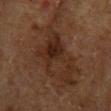<record>
  <biopsy_status>not biopsied; imaged during a skin examination</biopsy_status>
  <lighting>cross-polarized</lighting>
  <lesion_size>
    <long_diameter_mm_approx>11.5</long_diameter_mm_approx>
  </lesion_size>
  <site>chest</site>
  <patient>
    <sex>male</sex>
    <age_approx>65</age_approx>
  </patient>
  <automated_metrics>
    <vs_skin_darker_L>6.0</vs_skin_darker_L>
    <vs_skin_contrast_norm>7.0</vs_skin_contrast_norm>
    <border_irregularity_0_10>8.5</border_irregularity_0_10>
    <color_variation_0_10>5.5</color_variation_0_10>
  </automated_metrics>
  <image>
    <source>total-body photography crop</source>
    <field_of_view_mm>15</field_of_view_mm>
  </image>
</record>